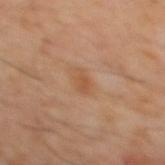Clinical impression:
The lesion was tiled from a total-body skin photograph and was not biopsied.
Image and clinical context:
The lesion is located on the mid back. Measured at roughly 2.5 mm in maximum diameter. Automated image analysis of the tile measured an area of roughly 3 mm² and a shape eccentricity near 0.85. The analysis additionally found a nevus-likeness score of about 0/100 and a lesion-detection confidence of about 100/100. A roughly 15 mm field-of-view crop from a total-body skin photograph. A male patient, aged around 60.